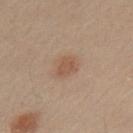The lesion was photographed on a routine skin check and not biopsied; there is no pathology result. The tile uses cross-polarized illumination. A male subject, aged approximately 50. From the left upper arm. Cropped from a whole-body photographic skin survey; the tile spans about 15 mm. Longest diameter approximately 2.5 mm.